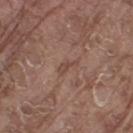Recorded during total-body skin imaging; not selected for excision or biopsy. A male patient aged 78–82. Approximately 2.5 mm at its widest. Automated tile analysis of the lesion measured a lesion area of about 2.5 mm², an outline eccentricity of about 0.9 (0 = round, 1 = elongated), and two-axis asymmetry of about 0.5. And it measured a border-irregularity index near 5/10 and a within-lesion color-variation index near 0/10. The software also gave a classifier nevus-likeness of about 0/100 and lesion-presence confidence of about 100/100. A 15 mm crop from a total-body photograph taken for skin-cancer surveillance. From the leg. Captured under white-light illumination.